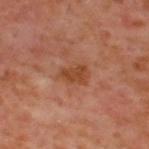The lesion was photographed on a routine skin check and not biopsied; there is no pathology result. Imaged with cross-polarized lighting. About 3.5 mm across. A lesion tile, about 15 mm wide, cut from a 3D total-body photograph. A male subject roughly 60 years of age. On the upper back.